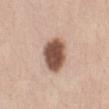subject: female, aged 33 to 37 | anatomic site: the front of the torso | tile lighting: white-light illumination | automated metrics: a lesion area of about 12 mm² and an outline eccentricity of about 0.65 (0 = round, 1 = elongated); about 20 CIELAB-L* units darker than the surrounding skin and a normalized lesion–skin contrast near 13; border irregularity of about 2 on a 0–10 scale | lesion diameter: ≈4.5 mm | image: total-body-photography crop, ~15 mm field of view.